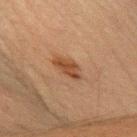biopsy status: total-body-photography surveillance lesion; no biopsy
anatomic site: the arm
tile lighting: cross-polarized illumination
subject: female, in their 60s
lesion size: about 3.5 mm
TBP lesion metrics: a classifier nevus-likeness of about 80/100 and a detector confidence of about 100 out of 100 that the crop contains a lesion
acquisition: ~15 mm tile from a whole-body skin photo